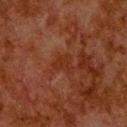The lesion was tiled from a total-body skin photograph and was not biopsied. Captured under cross-polarized illumination. The total-body-photography lesion software estimated an outline eccentricity of about 0.5 (0 = round, 1 = elongated) and two-axis asymmetry of about 0.35. And it measured a border-irregularity index near 3.5/10, a within-lesion color-variation index near 2/10, and a peripheral color-asymmetry measure near 1. The software also gave a nevus-likeness score of about 0/100 and a detector confidence of about 100 out of 100 that the crop contains a lesion. A male patient, aged around 80. Located on the upper back. Measured at roughly 2.5 mm in maximum diameter. Cropped from a total-body skin-imaging series; the visible field is about 15 mm.